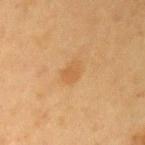{"biopsy_status": "not biopsied; imaged during a skin examination", "automated_metrics": {"cielab_L": 49, "cielab_a": 18, "cielab_b": 37, "vs_skin_darker_L": 6.0, "vs_skin_contrast_norm": 5.0, "color_variation_0_10": 1.5, "peripheral_color_asymmetry": 0.5, "nevus_likeness_0_100": 5, "lesion_detection_confidence_0_100": 100}, "image": {"source": "total-body photography crop", "field_of_view_mm": 15}, "patient": {"sex": "female", "age_approx": 55}, "site": "left upper arm", "lesion_size": {"long_diameter_mm_approx": 2.5}}